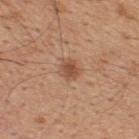Impression: Recorded during total-body skin imaging; not selected for excision or biopsy. Context: Automated tile analysis of the lesion measured a footprint of about 5 mm² and a shape eccentricity near 0.45. And it measured a lesion color around L≈51 a*≈22 b*≈31 in CIELAB and a lesion-to-skin contrast of about 7 (normalized; higher = more distinct). The analysis additionally found border irregularity of about 1.5 on a 0–10 scale and peripheral color asymmetry of about 1. This is a white-light tile. Located on the upper back. A 15 mm close-up tile from a total-body photography series done for melanoma screening. A male subject, aged around 45. Longest diameter approximately 2.5 mm.